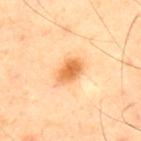| feature | finding |
|---|---|
| biopsy status | imaged on a skin check; not biopsied |
| lesion diameter | ≈3.5 mm |
| image | ~15 mm crop, total-body skin-cancer survey |
| subject | male, aged 38 to 42 |
| illumination | cross-polarized |
| image-analysis metrics | an average lesion color of about L≈70 a*≈27 b*≈48 (CIELAB), a lesion–skin lightness drop of about 15, and a normalized lesion–skin contrast near 9; radial color variation of about 1; a nevus-likeness score of about 100/100 |
| site | the upper back |